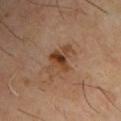The lesion was tiled from a total-body skin photograph and was not biopsied.
Automated tile analysis of the lesion measured a mean CIELAB color near L≈40 a*≈18 b*≈30 and roughly 8 lightness units darker than nearby skin. The analysis additionally found an automated nevus-likeness rating near 65 out of 100.
A region of skin cropped from a whole-body photographic capture, roughly 15 mm wide.
Located on the chest.
A male patient, aged 63 to 67.